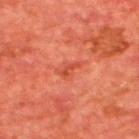Notes:
- notes · total-body-photography surveillance lesion; no biopsy
- lighting · cross-polarized
- patient · male, aged approximately 65
- anatomic site · the back
- lesion diameter · ≈2.5 mm
- image source · ~15 mm tile from a whole-body skin photo